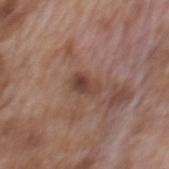A male subject, aged approximately 70. A 15 mm close-up tile from a total-body photography series done for melanoma screening. The lesion is on the mid back.